Cropped from a whole-body photographic skin survey; the tile spans about 15 mm · The lesion-visualizer software estimated a footprint of about 1.5 mm² and an eccentricity of roughly 0.65. It also reported an automated nevus-likeness rating near 0 out of 100 · the patient is a male in their 60s · the lesion is located on the front of the torso · about 1.5 mm across:
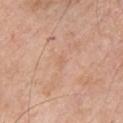| key | value |
|---|---|
| histopathology | a nodular basal cell carcinoma (malignant) |